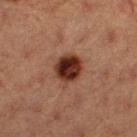Imaged during a routine full-body skin examination; the lesion was not biopsied and no histopathology is available.
Automated image analysis of the tile measured a lesion color around L≈26 a*≈20 b*≈24 in CIELAB, about 15 CIELAB-L* units darker than the surrounding skin, and a normalized border contrast of about 14.
A female subject, roughly 40 years of age.
The lesion's longest dimension is about 4 mm.
Cropped from a total-body skin-imaging series; the visible field is about 15 mm.
The lesion is located on the left thigh.